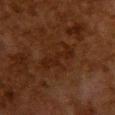This lesion was catalogued during total-body skin photography and was not selected for biopsy.
This image is a 15 mm lesion crop taken from a total-body photograph.
Imaged with cross-polarized lighting.
From the upper back.
A female patient, aged approximately 50.
Longest diameter approximately 5.5 mm.